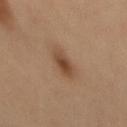This lesion was catalogued during total-body skin photography and was not selected for biopsy.
The total-body-photography lesion software estimated a shape eccentricity near 0.9. And it measured a mean CIELAB color near L≈44 a*≈18 b*≈30, about 10 CIELAB-L* units darker than the surrounding skin, and a normalized lesion–skin contrast near 8. And it measured a border-irregularity index near 3.5/10, a within-lesion color-variation index near 4/10, and radial color variation of about 1.
From the mid back.
The recorded lesion diameter is about 4 mm.
This is a cross-polarized tile.
A region of skin cropped from a whole-body photographic capture, roughly 15 mm wide.
A male patient, aged around 60.